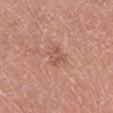Assessment: Captured during whole-body skin photography for melanoma surveillance; the lesion was not biopsied.